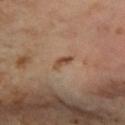{"biopsy_status": "not biopsied; imaged during a skin examination", "patient": {"sex": "female", "age_approx": 45}, "site": "right forearm", "image": {"source": "total-body photography crop", "field_of_view_mm": 15}, "automated_metrics": {"border_irregularity_0_10": 4.5, "peripheral_color_asymmetry": 0.0, "nevus_likeness_0_100": 0, "lesion_detection_confidence_0_100": 100}}